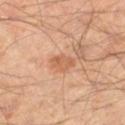{"biopsy_status": "not biopsied; imaged during a skin examination", "image": {"source": "total-body photography crop", "field_of_view_mm": 15}, "patient": {"sex": "male", "age_approx": 45}, "site": "right thigh"}